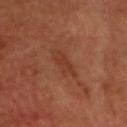The lesion was tiled from a total-body skin photograph and was not biopsied. From the head or neck. A 15 mm close-up extracted from a 3D total-body photography capture. A male subject in their mid- to late 60s. Imaged with cross-polarized lighting. The total-body-photography lesion software estimated an area of roughly 5.5 mm², a shape eccentricity near 0.85, and a shape-asymmetry score of about 0.35 (0 = symmetric). The software also gave about 6 CIELAB-L* units darker than the surrounding skin and a lesion-to-skin contrast of about 5 (normalized; higher = more distinct). And it measured a border-irregularity rating of about 4/10. And it measured lesion-presence confidence of about 100/100. The lesion's longest dimension is about 3.5 mm.